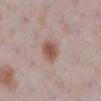Case summary:
• follow-up · catalogued during a skin exam; not biopsied
• image source · ~15 mm tile from a whole-body skin photo
• site · the right lower leg
• lighting · white-light illumination
• lesion size · ~3 mm (longest diameter)
• subject · female, in their 30s